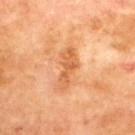The lesion was photographed on a routine skin check and not biopsied; there is no pathology result.
The subject is a male in their 70s.
An algorithmic analysis of the crop reported border irregularity of about 5 on a 0–10 scale, internal color variation of about 3 on a 0–10 scale, and peripheral color asymmetry of about 1.
Imaged with cross-polarized lighting.
Located on the upper back.
Approximately 5 mm at its widest.
A 15 mm crop from a total-body photograph taken for skin-cancer surveillance.